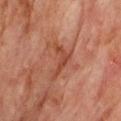workup = imaged on a skin check; not biopsied
body site = the upper back
subject = male, about 75 years old
acquisition = total-body-photography crop, ~15 mm field of view